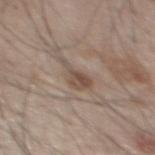Captured during whole-body skin photography for melanoma surveillance; the lesion was not biopsied.
A male subject, approximately 50 years of age.
Measured at roughly 3.5 mm in maximum diameter.
Automated image analysis of the tile measured a lesion color around L≈49 a*≈14 b*≈25 in CIELAB. And it measured a border-irregularity rating of about 6/10, internal color variation of about 4 on a 0–10 scale, and peripheral color asymmetry of about 1.5.
Located on the back.
Captured under white-light illumination.
Cropped from a total-body skin-imaging series; the visible field is about 15 mm.